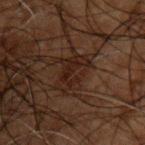Q: How was the tile lit?
A: cross-polarized illumination
Q: Patient demographics?
A: male, aged around 50
Q: What is the lesion's diameter?
A: ~4.5 mm (longest diameter)
Q: Where on the body is the lesion?
A: the upper back
Q: What kind of image is this?
A: ~15 mm crop, total-body skin-cancer survey